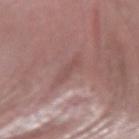notes: total-body-photography surveillance lesion; no biopsy | size: about 3.5 mm | location: the right forearm | illumination: white-light | acquisition: 15 mm crop, total-body photography | subject: female, about 65 years old.